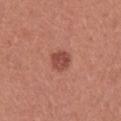biopsy status: total-body-photography surveillance lesion; no biopsy | subject: female, aged 23–27 | site: the left upper arm | size: ~3 mm (longest diameter) | acquisition: ~15 mm tile from a whole-body skin photo.